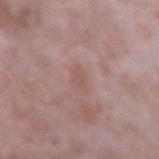Case summary:
• follow-up: no biopsy performed (imaged during a skin exam)
• location: the left forearm
• subject: male, aged approximately 65
• image source: total-body-photography crop, ~15 mm field of view